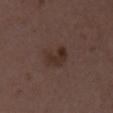lighting: white-light illumination
anatomic site: the chest
lesion diameter: about 3.5 mm
acquisition: ~15 mm crop, total-body skin-cancer survey
patient: female, roughly 50 years of age